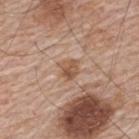<record>
<biopsy_status>not biopsied; imaged during a skin examination</biopsy_status>
<site>upper back</site>
<image>
  <source>total-body photography crop</source>
  <field_of_view_mm>15</field_of_view_mm>
</image>
<automated_metrics>
  <area_mm2_approx>4.5</area_mm2_approx>
  <eccentricity>0.35</eccentricity>
  <shape_asymmetry>0.3</shape_asymmetry>
  <cielab_L>54</cielab_L>
  <cielab_a>19</cielab_a>
  <cielab_b>31</cielab_b>
  <vs_skin_contrast_norm>7.0</vs_skin_contrast_norm>
</automated_metrics>
<lighting>white-light</lighting>
<patient>
  <sex>male</sex>
  <age_approx>65</age_approx>
</patient>
</record>